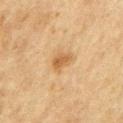biopsy_status: not biopsied; imaged during a skin examination
image:
  source: total-body photography crop
  field_of_view_mm: 15
lighting: cross-polarized
automated_metrics:
  nevus_likeness_0_100: 75
  lesion_detection_confidence_0_100: 100
site: chest
patient:
  sex: male
  age_approx: 75